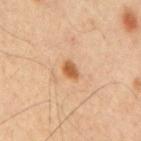  biopsy_status: not biopsied; imaged during a skin examination
  site: mid back
  patient:
    sex: male
    age_approx: 60
  lesion_size:
    long_diameter_mm_approx: 2.5
  automated_metrics:
    eccentricity: 0.75
    cielab_L: 57
    cielab_a: 23
    cielab_b: 38
    vs_skin_contrast_norm: 9.0
    peripheral_color_asymmetry: 0.5
  image:
    source: total-body photography crop
    field_of_view_mm: 15